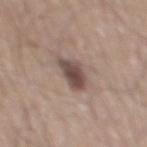follow-up: imaged on a skin check; not biopsied | body site: the mid back | tile lighting: white-light | TBP lesion metrics: a lesion color around L≈47 a*≈15 b*≈20 in CIELAB, roughly 14 lightness units darker than nearby skin, and a normalized lesion–skin contrast near 10.5; a border-irregularity rating of about 3/10, internal color variation of about 3.5 on a 0–10 scale, and peripheral color asymmetry of about 1 | subject: male, aged around 75 | image source: ~15 mm tile from a whole-body skin photo | lesion size: about 3.5 mm.